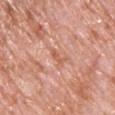Q: What is the imaging modality?
A: ~15 mm tile from a whole-body skin photo
Q: What is the anatomic site?
A: the back
Q: What lighting was used for the tile?
A: white-light illumination
Q: What are the patient's age and sex?
A: male, in their 80s
Q: Automated lesion metrics?
A: an area of roughly 2 mm², an outline eccentricity of about 0.95 (0 = round, 1 = elongated), and a symmetry-axis asymmetry near 0.45; a lesion color around L≈60 a*≈26 b*≈33 in CIELAB, a lesion–skin lightness drop of about 8, and a lesion-to-skin contrast of about 6 (normalized; higher = more distinct); a nevus-likeness score of about 0/100
Q: How large is the lesion?
A: about 2.5 mm